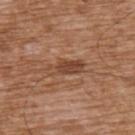Assessment: Captured during whole-body skin photography for melanoma surveillance; the lesion was not biopsied. Image and clinical context: Located on the upper back. The patient is a male aged approximately 75. A 15 mm crop from a total-body photograph taken for skin-cancer surveillance. The tile uses white-light illumination.